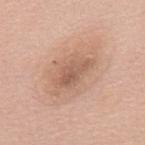| field | value |
|---|---|
| workup | total-body-photography surveillance lesion; no biopsy |
| subject | female, aged 58–62 |
| image source | ~15 mm tile from a whole-body skin photo |
| location | the mid back |